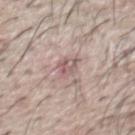No biopsy was performed on this lesion — it was imaged during a full skin examination and was not determined to be concerning. A roughly 15 mm field-of-view crop from a total-body skin photograph. A male patient, about 65 years old. The lesion is located on the chest.